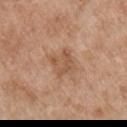• follow-up · imaged on a skin check; not biopsied
• acquisition · 15 mm crop, total-body photography
• patient · male, about 55 years old
• body site · the right upper arm